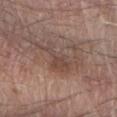Assessment:
The lesion was tiled from a total-body skin photograph and was not biopsied.
Background:
The lesion is on the right forearm. The lesion's longest dimension is about 7.5 mm. A region of skin cropped from a whole-body photographic capture, roughly 15 mm wide. The subject is a male aged 73–77.